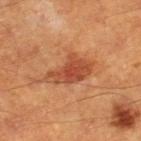Captured during whole-body skin photography for melanoma surveillance; the lesion was not biopsied. On the right lower leg. A 15 mm close-up extracted from a 3D total-body photography capture. A male patient about 60 years old. The tile uses cross-polarized illumination.